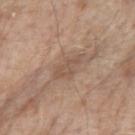On the right upper arm. A close-up tile cropped from a whole-body skin photograph, about 15 mm across. Imaged with white-light lighting. The lesion's longest dimension is about 4 mm. The patient is a female roughly 85 years of age or older.